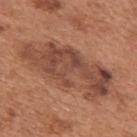Image and clinical context: Captured under white-light illumination. Automated image analysis of the tile measured a lesion area of about 34 mm², an eccentricity of roughly 0.95, and two-axis asymmetry of about 0.35. It also reported a detector confidence of about 90 out of 100 that the crop contains a lesion. On the back. Cropped from a total-body skin-imaging series; the visible field is about 15 mm. Longest diameter approximately 11 mm. A male patient aged 63 to 67.